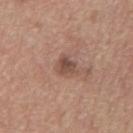Part of a total-body skin-imaging series; this lesion was reviewed on a skin check and was not flagged for biopsy. The subject is a male about 70 years old. The tile uses white-light illumination. A close-up tile cropped from a whole-body skin photograph, about 15 mm across. Located on the chest. Measured at roughly 2.5 mm in maximum diameter.